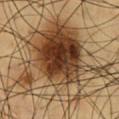Q: Was this lesion biopsied?
A: total-body-photography surveillance lesion; no biopsy
Q: What kind of image is this?
A: ~15 mm tile from a whole-body skin photo
Q: Automated lesion metrics?
A: a footprint of about 65 mm², an outline eccentricity of about 0.55 (0 = round, 1 = elongated), and a symmetry-axis asymmetry near 0.45; a mean CIELAB color near L≈43 a*≈17 b*≈33 and a lesion-to-skin contrast of about 12 (normalized; higher = more distinct); a peripheral color-asymmetry measure near 4; a nevus-likeness score of about 95/100 and a lesion-detection confidence of about 100/100
Q: How large is the lesion?
A: ≈11.5 mm
Q: What lighting was used for the tile?
A: cross-polarized
Q: Lesion location?
A: the chest
Q: Who is the patient?
A: male, aged approximately 60